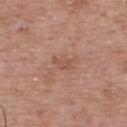Q: How was the tile lit?
A: white-light
Q: What kind of image is this?
A: ~15 mm crop, total-body skin-cancer survey
Q: Who is the patient?
A: male, roughly 65 years of age
Q: What is the lesion's diameter?
A: ~3 mm (longest diameter)
Q: Lesion location?
A: the upper back